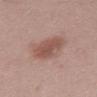{"biopsy_status": "not biopsied; imaged during a skin examination", "image": {"source": "total-body photography crop", "field_of_view_mm": 15}, "automated_metrics": {"cielab_L": 52, "cielab_a": 20, "cielab_b": 25, "vs_skin_darker_L": 10.0, "vs_skin_contrast_norm": 7.5, "border_irregularity_0_10": 3.0, "color_variation_0_10": 3.0, "peripheral_color_asymmetry": 1.0}, "lesion_size": {"long_diameter_mm_approx": 5.5}, "patient": {"sex": "female", "age_approx": 30}, "site": "left thigh"}